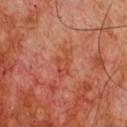No biopsy was performed on this lesion — it was imaged during a full skin examination and was not determined to be concerning. A 15 mm close-up tile from a total-body photography series done for melanoma screening. The tile uses cross-polarized illumination. The lesion is on the chest. A male subject about 55 years old.